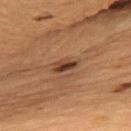Clinical impression:
Captured during whole-body skin photography for melanoma surveillance; the lesion was not biopsied.
Background:
The subject is a male aged approximately 85 or older. The lesion is located on the upper back. Cropped from a total-body skin-imaging series; the visible field is about 15 mm. The total-body-photography lesion software estimated a shape eccentricity near 0.9 and a symmetry-axis asymmetry near 0.3. The analysis additionally found border irregularity of about 3 on a 0–10 scale, a color-variation rating of about 3.5/10, and a peripheral color-asymmetry measure near 1.5. The tile uses cross-polarized illumination. The lesion's longest dimension is about 3 mm.